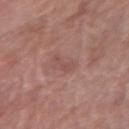No biopsy was performed on this lesion — it was imaged during a full skin examination and was not determined to be concerning. A female subject aged 58 to 62. A roughly 15 mm field-of-view crop from a total-body skin photograph. On the arm.